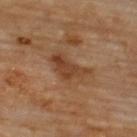Captured during whole-body skin photography for melanoma surveillance; the lesion was not biopsied. A male patient approximately 70 years of age. The lesion-visualizer software estimated an area of roughly 10 mm², an outline eccentricity of about 0.8 (0 = round, 1 = elongated), and a shape-asymmetry score of about 0.45 (0 = symmetric). It also reported a lesion color around L≈41 a*≈20 b*≈32 in CIELAB and a lesion–skin lightness drop of about 9. The analysis additionally found a color-variation rating of about 4/10. A roughly 15 mm field-of-view crop from a total-body skin photograph. From the back. The recorded lesion diameter is about 5 mm. Captured under cross-polarized illumination.